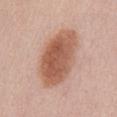The lesion was photographed on a routine skin check and not biopsied; there is no pathology result. On the abdomen. The patient is a male roughly 55 years of age. A 15 mm close-up extracted from a 3D total-body photography capture.